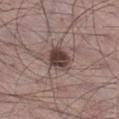– biopsy status — catalogued during a skin exam; not biopsied
– image — 15 mm crop, total-body photography
– patient — male, in their mid- to late 50s
– anatomic site — the right lower leg
– lighting — white-light